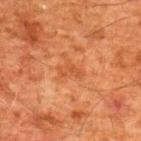The lesion was tiled from a total-body skin photograph and was not biopsied.
The lesion is located on the upper back.
Longest diameter approximately 3 mm.
An algorithmic analysis of the crop reported an area of roughly 3 mm², a shape eccentricity near 0.8, and a symmetry-axis asymmetry near 0.5. It also reported a lesion–skin lightness drop of about 6 and a lesion-to-skin contrast of about 5 (normalized; higher = more distinct).
A close-up tile cropped from a whole-body skin photograph, about 15 mm across.
The patient is a male approximately 60 years of age.
Imaged with cross-polarized lighting.